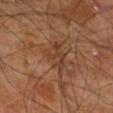No biopsy was performed on this lesion — it was imaged during a full skin examination and was not determined to be concerning.
A region of skin cropped from a whole-body photographic capture, roughly 15 mm wide.
The lesion's longest dimension is about 5 mm.
The lesion is on the right leg.
A male subject in their 60s.